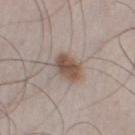The lesion's longest dimension is about 3.5 mm.
Located on the left thigh.
The patient is a male aged 48 to 52.
An algorithmic analysis of the crop reported an automated nevus-likeness rating near 100 out of 100.
A region of skin cropped from a whole-body photographic capture, roughly 15 mm wide.
Imaged with white-light lighting.